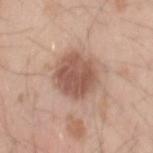Impression: Captured during whole-body skin photography for melanoma surveillance; the lesion was not biopsied. Image and clinical context: A close-up tile cropped from a whole-body skin photograph, about 15 mm across. The recorded lesion diameter is about 5 mm. Automated tile analysis of the lesion measured a shape eccentricity near 0.5 and a shape-asymmetry score of about 0.15 (0 = symmetric). The patient is a male aged around 20. On the left forearm.